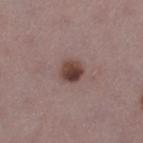Assessment:
Imaged during a routine full-body skin examination; the lesion was not biopsied and no histopathology is available.
Clinical summary:
On the leg. The subject is a female in their mid- to late 40s. The total-body-photography lesion software estimated an area of roughly 5.5 mm² and two-axis asymmetry of about 0.2. It also reported a border-irregularity rating of about 1.5/10, a within-lesion color-variation index near 5.5/10, and a peripheral color-asymmetry measure near 2. The software also gave a nevus-likeness score of about 95/100 and a detector confidence of about 100 out of 100 that the crop contains a lesion. The recorded lesion diameter is about 2.5 mm. Captured under white-light illumination. A close-up tile cropped from a whole-body skin photograph, about 15 mm across.